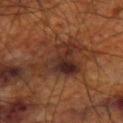No biopsy was performed on this lesion — it was imaged during a full skin examination and was not determined to be concerning.
A male subject, in their 70s.
Captured under cross-polarized illumination.
The lesion-visualizer software estimated an average lesion color of about L≈29 a*≈20 b*≈25 (CIELAB) and a normalized lesion–skin contrast near 10.5. The software also gave a border-irregularity index near 7/10 and a peripheral color-asymmetry measure near 3.
From the right thigh.
Cropped from a whole-body photographic skin survey; the tile spans about 15 mm.
The recorded lesion diameter is about 7 mm.